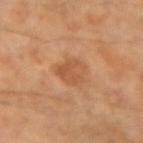Captured during whole-body skin photography for melanoma surveillance; the lesion was not biopsied.
This image is a 15 mm lesion crop taken from a total-body photograph.
The lesion is on the left forearm.
The lesion's longest dimension is about 3.5 mm.
The subject is a female aged 58 to 62.
Captured under cross-polarized illumination.